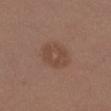Captured during whole-body skin photography for melanoma surveillance; the lesion was not biopsied. A roughly 15 mm field-of-view crop from a total-body skin photograph. A female patient, aged 38–42. The total-body-photography lesion software estimated a border-irregularity index near 1/10, a color-variation rating of about 2.5/10, and peripheral color asymmetry of about 1. And it measured an automated nevus-likeness rating near 5 out of 100. The lesion is on the leg. The lesion's longest dimension is about 4.5 mm. The tile uses white-light illumination.